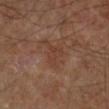notes=total-body-photography surveillance lesion; no biopsy | patient=approximately 65 years of age | imaging modality=total-body-photography crop, ~15 mm field of view | location=the left lower leg.